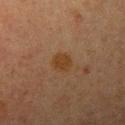| field | value |
|---|---|
| biopsy status | total-body-photography surveillance lesion; no biopsy |
| image-analysis metrics | a mean CIELAB color near L≈31 a*≈16 b*≈29 and roughly 5 lightness units darker than nearby skin |
| location | the left upper arm |
| image | 15 mm crop, total-body photography |
| subject | female, approximately 55 years of age |
| lighting | cross-polarized |
| diameter | ≈2.5 mm |